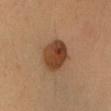follow-up: no biopsy performed (imaged during a skin exam) | anatomic site: the head or neck | TBP lesion metrics: a lesion area of about 12 mm², a shape eccentricity near 0.6, and a shape-asymmetry score of about 0.1 (0 = symmetric); a lesion color around L≈42 a*≈21 b*≈33 in CIELAB, roughly 14 lightness units darker than nearby skin, and a lesion-to-skin contrast of about 10.5 (normalized; higher = more distinct) | size: ≈4 mm | subject: female, aged approximately 35 | image source: total-body-photography crop, ~15 mm field of view | tile lighting: cross-polarized illumination.